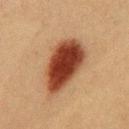workup: catalogued during a skin exam; not biopsied
image-analysis metrics: a border-irregularity index near 2.5/10 and internal color variation of about 5.5 on a 0–10 scale; an automated nevus-likeness rating near 100 out of 100 and a detector confidence of about 100 out of 100 that the crop contains a lesion
imaging modality: ~15 mm tile from a whole-body skin photo
patient: male, aged 38 to 42
tile lighting: cross-polarized
lesion diameter: ~7 mm (longest diameter)
body site: the chest